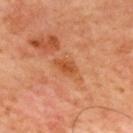No biopsy was performed on this lesion — it was imaged during a full skin examination and was not determined to be concerning. A 15 mm close-up tile from a total-body photography series done for melanoma screening. Located on the mid back. A subject roughly 65 years of age. About 3 mm across. Captured under cross-polarized illumination. Automated tile analysis of the lesion measured a lesion color around L≈52 a*≈29 b*≈41 in CIELAB and a lesion-to-skin contrast of about 7 (normalized; higher = more distinct). The analysis additionally found a classifier nevus-likeness of about 0/100 and lesion-presence confidence of about 100/100.